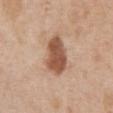The lesion was tiled from a total-body skin photograph and was not biopsied.